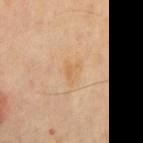Case summary:
* follow-up · catalogued during a skin exam; not biopsied
* acquisition · ~15 mm crop, total-body skin-cancer survey
* illumination · cross-polarized
* body site · the mid back
* subject · male, in their mid- to late 40s